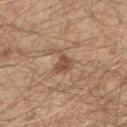Clinical impression:
The lesion was tiled from a total-body skin photograph and was not biopsied.
Background:
Imaged with white-light lighting. A 15 mm close-up tile from a total-body photography series done for melanoma screening. Located on the right forearm. A male subject aged 33–37. Approximately 3 mm at its widest. The lesion-visualizer software estimated a lesion color around L≈51 a*≈19 b*≈28 in CIELAB, a lesion–skin lightness drop of about 10, and a lesion-to-skin contrast of about 7 (normalized; higher = more distinct). The analysis additionally found an automated nevus-likeness rating near 15 out of 100 and lesion-presence confidence of about 100/100.